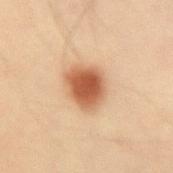workup = catalogued during a skin exam; not biopsied
patient = male, aged around 35
acquisition = 15 mm crop, total-body photography
location = the left forearm
image-analysis metrics = a border-irregularity index near 2/10, a color-variation rating of about 3.5/10, and peripheral color asymmetry of about 0.5
lesion size = about 4.5 mm
tile lighting = cross-polarized illumination